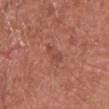Q: Was this lesion biopsied?
A: total-body-photography surveillance lesion; no biopsy
Q: How large is the lesion?
A: ~3.5 mm (longest diameter)
Q: What kind of image is this?
A: 15 mm crop, total-body photography
Q: What is the anatomic site?
A: the chest
Q: Automated lesion metrics?
A: an area of roughly 3 mm² and an outline eccentricity of about 0.95 (0 = round, 1 = elongated)
Q: Illumination type?
A: white-light illumination
Q: Who is the patient?
A: male, aged 73–77